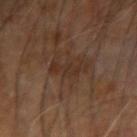biopsy_status: not biopsied; imaged during a skin examination
lighting: cross-polarized
site: right arm
patient:
  sex: male
  age_approx: 60
lesion_size:
  long_diameter_mm_approx: 4.0
image:
  source: total-body photography crop
  field_of_view_mm: 15